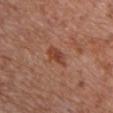Impression:
This lesion was catalogued during total-body skin photography and was not selected for biopsy.
Context:
From the chest. Imaged with white-light lighting. A male patient, in their mid- to late 60s. Longest diameter approximately 3 mm. Cropped from a total-body skin-imaging series; the visible field is about 15 mm.